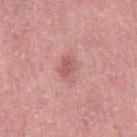Case summary:
- location: the right thigh
- acquisition: ~15 mm crop, total-body skin-cancer survey
- subject: female, aged 53–57
- lighting: white-light illumination